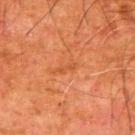Imaged during a routine full-body skin examination; the lesion was not biopsied and no histopathology is available. The recorded lesion diameter is about 2.5 mm. A region of skin cropped from a whole-body photographic capture, roughly 15 mm wide. A male patient aged around 80. The lesion is on the left upper arm. The lesion-visualizer software estimated an automated nevus-likeness rating near 0 out of 100. This is a cross-polarized tile.